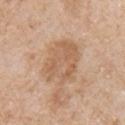Recorded during total-body skin imaging; not selected for excision or biopsy. From the chest. The lesion-visualizer software estimated a mean CIELAB color near L≈60 a*≈19 b*≈33, about 8 CIELAB-L* units darker than the surrounding skin, and a lesion-to-skin contrast of about 6 (normalized; higher = more distinct). A male patient, aged 63 to 67. A 15 mm crop from a total-body photograph taken for skin-cancer surveillance.